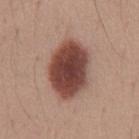Impression:
The lesion was photographed on a routine skin check and not biopsied; there is no pathology result.
Clinical summary:
Captured under white-light illumination. From the abdomen. A male subject aged 28 to 32. Longest diameter approximately 7 mm. A 15 mm crop from a total-body photograph taken for skin-cancer surveillance. The total-body-photography lesion software estimated an area of roughly 25 mm², an outline eccentricity of about 0.75 (0 = round, 1 = elongated), and a shape-asymmetry score of about 0.1 (0 = symmetric). And it measured a lesion color around L≈44 a*≈22 b*≈25 in CIELAB, roughly 18 lightness units darker than nearby skin, and a normalized border contrast of about 13. The software also gave a nevus-likeness score of about 100/100 and a lesion-detection confidence of about 100/100.